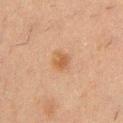The tile uses cross-polarized illumination. A 15 mm crop from a total-body photograph taken for skin-cancer surveillance. The lesion is on the chest. A male patient, approximately 50 years of age.